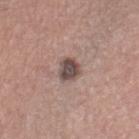Case summary:
* workup · total-body-photography surveillance lesion; no biopsy
* tile lighting · white-light
* patient · female, approximately 55 years of age
* body site · the right thigh
* size · ~3 mm (longest diameter)
* image source · 15 mm crop, total-body photography